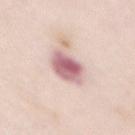notes: catalogued during a skin exam; not biopsied | image source: 15 mm crop, total-body photography | body site: the chest | TBP lesion metrics: a lesion color around L≈64 a*≈23 b*≈18 in CIELAB and a lesion-to-skin contrast of about 10 (normalized; higher = more distinct); an automated nevus-likeness rating near 15 out of 100 and lesion-presence confidence of about 100/100 | lighting: white-light | lesion diameter: ≈4 mm | subject: female, aged around 65.